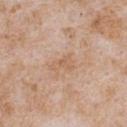<record>
<site>chest</site>
<patient>
  <sex>male</sex>
  <age_approx>65</age_approx>
</patient>
<image>
  <source>total-body photography crop</source>
  <field_of_view_mm>15</field_of_view_mm>
</image>
</record>